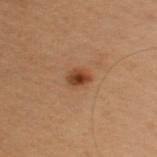workup — imaged on a skin check; not biopsied | location — the back | lesion size — ~3 mm (longest diameter) | image-analysis metrics — an area of roughly 4 mm² and an outline eccentricity of about 0.8 (0 = round, 1 = elongated); a color-variation rating of about 3.5/10 and peripheral color asymmetry of about 1; a classifier nevus-likeness of about 100/100 and a lesion-detection confidence of about 100/100 | patient — female, about 50 years old | tile lighting — cross-polarized | imaging modality — ~15 mm crop, total-body skin-cancer survey.